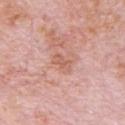Impression: Recorded during total-body skin imaging; not selected for excision or biopsy. Acquisition and patient details: A 15 mm close-up extracted from a 3D total-body photography capture. A male subject aged around 80. Imaged with white-light lighting. Measured at roughly 2.5 mm in maximum diameter. The lesion is located on the chest.